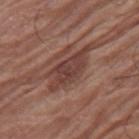• biopsy status · total-body-photography surveillance lesion; no biopsy
• location · the right thigh
• subject · female, in their 80s
• image · total-body-photography crop, ~15 mm field of view
• tile lighting · white-light
• diameter · about 7 mm
• automated metrics · an area of roughly 12 mm² and an eccentricity of roughly 0.9; a within-lesion color-variation index near 3.5/10 and a peripheral color-asymmetry measure near 1